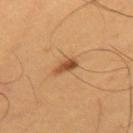Located on the mid back. The lesion-visualizer software estimated a shape eccentricity near 0.85 and a symmetry-axis asymmetry near 0.25. And it measured lesion-presence confidence of about 100/100. Captured under cross-polarized illumination. A roughly 15 mm field-of-view crop from a total-body skin photograph. The subject is a male aged 53–57.